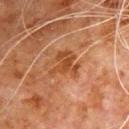{
  "biopsy_status": "not biopsied; imaged during a skin examination",
  "lighting": "cross-polarized",
  "patient": {
    "sex": "male",
    "age_approx": 80
  },
  "image": {
    "source": "total-body photography crop",
    "field_of_view_mm": 15
  },
  "automated_metrics": {
    "area_mm2_approx": 9.0,
    "shape_asymmetry": 0.45,
    "border_irregularity_0_10": 6.5,
    "color_variation_0_10": 3.0,
    "peripheral_color_asymmetry": 1.0
  },
  "site": "chest"
}